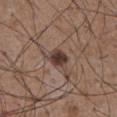* workup · imaged on a skin check; not biopsied
* anatomic site · the abdomen
* diameter · ≈3 mm
* subject · male, aged 53–57
* imaging modality · total-body-photography crop, ~15 mm field of view
* illumination · white-light
* image-analysis metrics · border irregularity of about 3 on a 0–10 scale, a color-variation rating of about 3.5/10, and peripheral color asymmetry of about 1